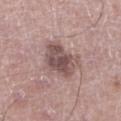Assessment:
Imaged during a routine full-body skin examination; the lesion was not biopsied and no histopathology is available.
Image and clinical context:
Captured under white-light illumination. A 15 mm close-up tile from a total-body photography series done for melanoma screening. On the left lower leg. A male patient, roughly 65 years of age. The lesion-visualizer software estimated an area of roughly 15 mm² and a symmetry-axis asymmetry near 0.25. The analysis additionally found a border-irregularity index near 3/10, a color-variation rating of about 5/10, and a peripheral color-asymmetry measure near 1.5. It also reported a classifier nevus-likeness of about 30/100 and a lesion-detection confidence of about 100/100. Measured at roughly 5 mm in maximum diameter.